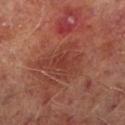Assessment:
Recorded during total-body skin imaging; not selected for excision or biopsy.
Background:
A male subject, aged approximately 65. Located on the left lower leg. Automated image analysis of the tile measured an eccentricity of roughly 0.6 and a symmetry-axis asymmetry near 0.35. The software also gave a lesion color around L≈39 a*≈26 b*≈27 in CIELAB, about 6 CIELAB-L* units darker than the surrounding skin, and a normalized lesion–skin contrast near 5. And it measured a nevus-likeness score of about 0/100 and a detector confidence of about 100 out of 100 that the crop contains a lesion. The recorded lesion diameter is about 6 mm. Cropped from a whole-body photographic skin survey; the tile spans about 15 mm.